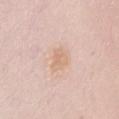Q: Is there a histopathology result?
A: total-body-photography surveillance lesion; no biopsy
Q: How large is the lesion?
A: ~3 mm (longest diameter)
Q: Automated lesion metrics?
A: border irregularity of about 2.5 on a 0–10 scale and radial color variation of about 1
Q: Where on the body is the lesion?
A: the chest
Q: What are the patient's age and sex?
A: female, approximately 45 years of age
Q: How was this image acquired?
A: ~15 mm crop, total-body skin-cancer survey
Q: Illumination type?
A: white-light illumination